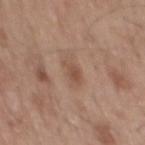Captured during whole-body skin photography for melanoma surveillance; the lesion was not biopsied. Imaged with white-light lighting. The lesion-visualizer software estimated a border-irregularity index near 4/10 and internal color variation of about 3 on a 0–10 scale. The lesion is on the back. The patient is a male aged around 55. Cropped from a whole-body photographic skin survey; the tile spans about 15 mm.